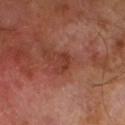Approximately 3 mm at its widest. Captured under cross-polarized illumination. A 15 mm close-up tile from a total-body photography series done for melanoma screening. The patient is a male aged approximately 65. The total-body-photography lesion software estimated a classifier nevus-likeness of about 0/100 and lesion-presence confidence of about 100/100.